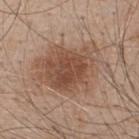Q: Is there a histopathology result?
A: no biopsy performed (imaged during a skin exam)